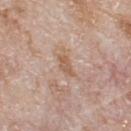Part of a total-body skin-imaging series; this lesion was reviewed on a skin check and was not flagged for biopsy.
On the upper back.
The recorded lesion diameter is about 3 mm.
This image is a 15 mm lesion crop taken from a total-body photograph.
A male subject approximately 80 years of age.
The tile uses white-light illumination.